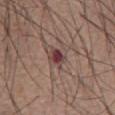follow-up = imaged on a skin check; not biopsied | image source = ~15 mm tile from a whole-body skin photo | lesion size = ~2.5 mm (longest diameter) | tile lighting = white-light illumination | site = the abdomen | patient = male, in their mid-50s.